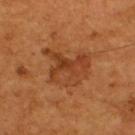Clinical impression:
The lesion was tiled from a total-body skin photograph and was not biopsied.
Clinical summary:
The total-body-photography lesion software estimated a shape eccentricity near 0.55 and a symmetry-axis asymmetry near 0.35. It also reported about 7 CIELAB-L* units darker than the surrounding skin and a lesion-to-skin contrast of about 6.5 (normalized; higher = more distinct). And it measured a border-irregularity rating of about 4.5/10, a within-lesion color-variation index near 5/10, and radial color variation of about 2. Imaged with cross-polarized lighting. Cropped from a total-body skin-imaging series; the visible field is about 15 mm. The lesion is on the upper back. The patient is a male in their mid- to late 50s.